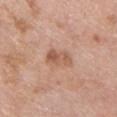Q: Was a biopsy performed?
A: imaged on a skin check; not biopsied
Q: Illumination type?
A: white-light
Q: How large is the lesion?
A: ≈3 mm
Q: Where on the body is the lesion?
A: the chest
Q: What are the patient's age and sex?
A: male, in their 60s
Q: What is the imaging modality?
A: 15 mm crop, total-body photography
Q: What did automated image analysis measure?
A: an area of roughly 5.5 mm², a shape eccentricity near 0.8, and a symmetry-axis asymmetry near 0.3; an average lesion color of about L≈57 a*≈22 b*≈30 (CIELAB), a lesion–skin lightness drop of about 10, and a normalized lesion–skin contrast near 6.5